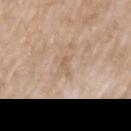Assessment: No biopsy was performed on this lesion — it was imaged during a full skin examination and was not determined to be concerning. Background: This image is a 15 mm lesion crop taken from a total-body photograph. This is a white-light tile. The lesion is located on the left upper arm. The recorded lesion diameter is about 2.5 mm. Automated image analysis of the tile measured a footprint of about 2 mm² and an outline eccentricity of about 0.95 (0 = round, 1 = elongated). And it measured an automated nevus-likeness rating near 0 out of 100 and a lesion-detection confidence of about 95/100. The subject is a female in their mid-70s.